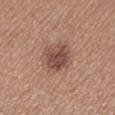Clinical impression: Imaged during a routine full-body skin examination; the lesion was not biopsied and no histopathology is available. Acquisition and patient details: The patient is a female aged around 35. The lesion is on the left lower leg. The tile uses white-light illumination. A 15 mm close-up extracted from a 3D total-body photography capture. The total-body-photography lesion software estimated an outline eccentricity of about 0.65 (0 = round, 1 = elongated) and a symmetry-axis asymmetry near 0.15. The analysis additionally found an average lesion color of about L≈48 a*≈20 b*≈25 (CIELAB), roughly 11 lightness units darker than nearby skin, and a normalized border contrast of about 8.5. The analysis additionally found a peripheral color-asymmetry measure near 1.5. And it measured a lesion-detection confidence of about 100/100.